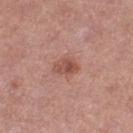Assessment:
Part of a total-body skin-imaging series; this lesion was reviewed on a skin check and was not flagged for biopsy.